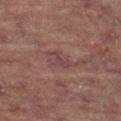notes: imaged on a skin check; not biopsied
image-analysis metrics: a footprint of about 3 mm² and an outline eccentricity of about 0.8 (0 = round, 1 = elongated); about 5 CIELAB-L* units darker than the surrounding skin and a normalized border contrast of about 6; a border-irregularity rating of about 7/10 and internal color variation of about 0 on a 0–10 scale; a classifier nevus-likeness of about 0/100
patient: female, aged 68 to 72
diameter: about 3 mm
imaging modality: ~15 mm tile from a whole-body skin photo
site: the leg